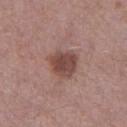notes: catalogued during a skin exam; not biopsied.